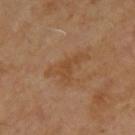biopsy_status: not biopsied; imaged during a skin examination
automated_metrics:
  border_irregularity_0_10: 8.0
  color_variation_0_10: 1.5
  peripheral_color_asymmetry: 0.5
  lesion_detection_confidence_0_100: 100
lesion_size:
  long_diameter_mm_approx: 5.0
patient:
  sex: male
  age_approx: 70
lighting: cross-polarized
site: upper back
image:
  source: total-body photography crop
  field_of_view_mm: 15